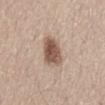{
  "biopsy_status": "not biopsied; imaged during a skin examination",
  "lighting": "white-light",
  "patient": {
    "sex": "female",
    "age_approx": 65
  },
  "site": "back",
  "automated_metrics": {
    "color_variation_0_10": 5.0
  },
  "image": {
    "source": "total-body photography crop",
    "field_of_view_mm": 15
  },
  "lesion_size": {
    "long_diameter_mm_approx": 3.5
  }
}